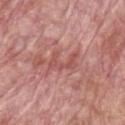The lesion was photographed on a routine skin check and not biopsied; there is no pathology result. The total-body-photography lesion software estimated border irregularity of about 8.5 on a 0–10 scale and radial color variation of about 0. The software also gave a nevus-likeness score of about 0/100 and a detector confidence of about 65 out of 100 that the crop contains a lesion. A male patient aged 63–67. A region of skin cropped from a whole-body photographic capture, roughly 15 mm wide. Measured at roughly 3.5 mm in maximum diameter. The lesion is located on the chest. The tile uses white-light illumination.